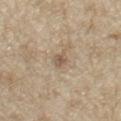Clinical impression: Captured during whole-body skin photography for melanoma surveillance; the lesion was not biopsied. Clinical summary: Cropped from a whole-body photographic skin survey; the tile spans about 15 mm. A male patient, aged approximately 70. The lesion is on the left upper arm.